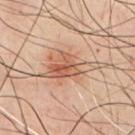Impression:
Part of a total-body skin-imaging series; this lesion was reviewed on a skin check and was not flagged for biopsy.
Context:
On the chest. The lesion's longest dimension is about 7 mm. A 15 mm close-up extracted from a 3D total-body photography capture. Automated tile analysis of the lesion measured a lesion area of about 14 mm² and an eccentricity of roughly 0.85. The analysis additionally found a mean CIELAB color near L≈58 a*≈21 b*≈31, roughly 11 lightness units darker than nearby skin, and a normalized border contrast of about 7. The software also gave a within-lesion color-variation index near 8/10 and peripheral color asymmetry of about 3. This is a cross-polarized tile. A male subject approximately 50 years of age.